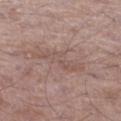Impression: Part of a total-body skin-imaging series; this lesion was reviewed on a skin check and was not flagged for biopsy. Clinical summary: The lesion-visualizer software estimated a color-variation rating of about 3/10. And it measured a classifier nevus-likeness of about 0/100. A male subject, approximately 50 years of age. The lesion is located on the leg. The recorded lesion diameter is about 6 mm. A close-up tile cropped from a whole-body skin photograph, about 15 mm across. Imaged with white-light lighting.